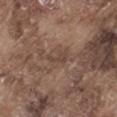The lesion was photographed on a routine skin check and not biopsied; there is no pathology result.
This is a white-light tile.
A male patient, aged 73–77.
Longest diameter approximately 3 mm.
Cropped from a whole-body photographic skin survey; the tile spans about 15 mm.
From the left thigh.